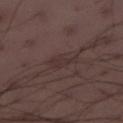diameter — about 3.5 mm | site — the right lower leg | patient — male, about 40 years old | image source — 15 mm crop, total-body photography.